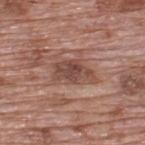Assessment: Recorded during total-body skin imaging; not selected for excision or biopsy. Acquisition and patient details: Longest diameter approximately 4.5 mm. A male subject in their 70s. Imaged with white-light lighting. The total-body-photography lesion software estimated an average lesion color of about L≈46 a*≈20 b*≈25 (CIELAB), a lesion–skin lightness drop of about 10, and a lesion-to-skin contrast of about 8 (normalized; higher = more distinct). The analysis additionally found a nevus-likeness score of about 0/100 and lesion-presence confidence of about 95/100. A 15 mm close-up extracted from a 3D total-body photography capture. Located on the upper back.